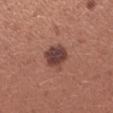Part of a total-body skin-imaging series; this lesion was reviewed on a skin check and was not flagged for biopsy.
The patient is a female aged approximately 25.
A close-up tile cropped from a whole-body skin photograph, about 15 mm across.
From the left forearm.